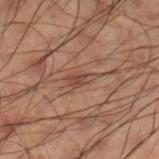Recorded during total-body skin imaging; not selected for excision or biopsy.
The subject is a male about 50 years old.
Automated tile analysis of the lesion measured an area of roughly 3.5 mm², a shape eccentricity near 0.85, and a symmetry-axis asymmetry near 0.4. The analysis additionally found an average lesion color of about L≈33 a*≈16 b*≈21 (CIELAB). It also reported a classifier nevus-likeness of about 0/100 and lesion-presence confidence of about 90/100.
From the right lower leg.
A roughly 15 mm field-of-view crop from a total-body skin photograph.
Imaged with cross-polarized lighting.